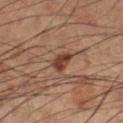{
  "biopsy_status": "not biopsied; imaged during a skin examination",
  "image": {
    "source": "total-body photography crop",
    "field_of_view_mm": 15
  },
  "lighting": "cross-polarized",
  "patient": {
    "sex": "male",
    "age_approx": 50
  },
  "site": "left lower leg",
  "automated_metrics": {
    "area_mm2_approx": 4.5,
    "eccentricity": 0.75,
    "cielab_L": 30,
    "cielab_a": 17,
    "cielab_b": 23,
    "vs_skin_darker_L": 9.0,
    "vs_skin_contrast_norm": 9.0,
    "border_irregularity_0_10": 2.5,
    "color_variation_0_10": 2.0,
    "peripheral_color_asymmetry": 1.0
  }
}